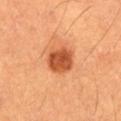- patient: male, roughly 60 years of age
- acquisition: total-body-photography crop, ~15 mm field of view
- lesion diameter: ~3.5 mm (longest diameter)
- tile lighting: cross-polarized
- automated lesion analysis: a lesion color around L≈49 a*≈30 b*≈39 in CIELAB, roughly 14 lightness units darker than nearby skin, and a normalized lesion–skin contrast near 9.5; an automated nevus-likeness rating near 100 out of 100 and a detector confidence of about 100 out of 100 that the crop contains a lesion
- body site: the right thigh